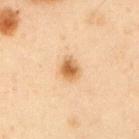Assessment: No biopsy was performed on this lesion — it was imaged during a full skin examination and was not determined to be concerning. Context: From the left upper arm. A male subject, about 55 years old. A 15 mm close-up tile from a total-body photography series done for melanoma screening. The recorded lesion diameter is about 2.5 mm. This is a cross-polarized tile.